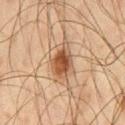Assessment: The lesion was tiled from a total-body skin photograph and was not biopsied. Context: Imaged with cross-polarized lighting. A male subject aged 43 to 47. From the left thigh. The total-body-photography lesion software estimated an area of roughly 10 mm², a shape eccentricity near 0.8, and a symmetry-axis asymmetry near 0.25. The analysis additionally found a border-irregularity rating of about 3/10, a within-lesion color-variation index near 5.5/10, and a peripheral color-asymmetry measure near 1.5. The analysis additionally found a nevus-likeness score of about 95/100 and a lesion-detection confidence of about 100/100. The lesion's longest dimension is about 4.5 mm. A 15 mm crop from a total-body photograph taken for skin-cancer surveillance.